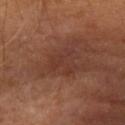notes = no biopsy performed (imaged during a skin exam); location = the left upper arm; acquisition = ~15 mm crop, total-body skin-cancer survey; patient = male, about 65 years old.